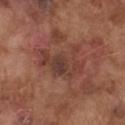Recorded during total-body skin imaging; not selected for excision or biopsy. From the chest. The total-body-photography lesion software estimated a within-lesion color-variation index near 5.5/10 and peripheral color asymmetry of about 1.5. A 15 mm close-up extracted from a 3D total-body photography capture. The tile uses white-light illumination. A male subject, in their mid-70s.